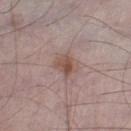follow-up — catalogued during a skin exam; not biopsied
TBP lesion metrics — an outline eccentricity of about 0.55 (0 = round, 1 = elongated) and a shape-asymmetry score of about 0.25 (0 = symmetric)
size — ≈2.5 mm
image source — ~15 mm crop, total-body skin-cancer survey
tile lighting — white-light illumination
patient — male, in their 70s
body site — the leg